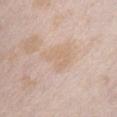| field | value |
|---|---|
| lighting | white-light illumination |
| anatomic site | the chest |
| size | ≈4 mm |
| imaging modality | ~15 mm crop, total-body skin-cancer survey |
| subject | female, aged approximately 25 |
| automated metrics | a lesion area of about 7.5 mm², an outline eccentricity of about 0.6 (0 = round, 1 = elongated), and a symmetry-axis asymmetry near 0.5; a mean CIELAB color near L≈67 a*≈15 b*≈30, a lesion–skin lightness drop of about 5, and a normalized lesion–skin contrast near 4.5; a nevus-likeness score of about 0/100 and a lesion-detection confidence of about 100/100 |